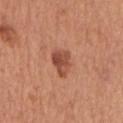This lesion was catalogued during total-body skin photography and was not selected for biopsy. A male subject approximately 65 years of age. This image is a 15 mm lesion crop taken from a total-body photograph. Approximately 3.5 mm at its widest. An algorithmic analysis of the crop reported an average lesion color of about L≈49 a*≈27 b*≈32 (CIELAB), about 12 CIELAB-L* units darker than the surrounding skin, and a normalized border contrast of about 8.5. On the mid back. The tile uses white-light illumination.